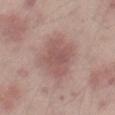<record>
<biopsy_status>not biopsied; imaged during a skin examination</biopsy_status>
<patient>
  <sex>male</sex>
  <age_approx>65</age_approx>
</patient>
<site>right thigh</site>
<lesion_size>
  <long_diameter_mm_approx>5.0</long_diameter_mm_approx>
</lesion_size>
<image>
  <source>total-body photography crop</source>
  <field_of_view_mm>15</field_of_view_mm>
</image>
<lighting>white-light</lighting>
</record>